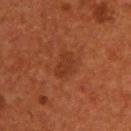<record>
  <patient>
    <sex>male</sex>
    <age_approx>55</age_approx>
  </patient>
  <site>upper back</site>
  <image>
    <source>total-body photography crop</source>
    <field_of_view_mm>15</field_of_view_mm>
  </image>
  <lighting>cross-polarized</lighting>
  <automated_metrics>
    <area_mm2_approx>5.5</area_mm2_approx>
    <eccentricity>0.75</eccentricity>
    <shape_asymmetry>0.3</shape_asymmetry>
    <nevus_likeness_0_100>0</nevus_likeness_0_100>
    <lesion_detection_confidence_0_100>100</lesion_detection_confidence_0_100>
  </automated_metrics>
</record>